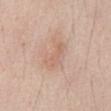Q: What are the patient's age and sex?
A: male, approximately 55 years of age
Q: Lesion size?
A: ≈4 mm
Q: Automated lesion metrics?
A: a footprint of about 9 mm², an eccentricity of roughly 0.8, and a symmetry-axis asymmetry near 0.3; roughly 7 lightness units darker than nearby skin and a normalized lesion–skin contrast near 4.5; a border-irregularity rating of about 4/10 and a within-lesion color-variation index near 2.5/10; an automated nevus-likeness rating near 20 out of 100 and a detector confidence of about 100 out of 100 that the crop contains a lesion
Q: How was this image acquired?
A: ~15 mm tile from a whole-body skin photo
Q: Illumination type?
A: white-light illumination
Q: What is the anatomic site?
A: the abdomen